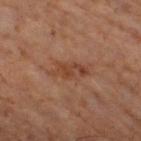biopsy status = catalogued during a skin exam; not biopsied | site = the right thigh | subject = male, aged 58–62 | tile lighting = cross-polarized illumination | image = ~15 mm crop, total-body skin-cancer survey | lesion diameter = ~4 mm (longest diameter).